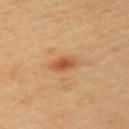Assessment:
Imaged during a routine full-body skin examination; the lesion was not biopsied and no histopathology is available.
Clinical summary:
A male subject roughly 40 years of age. On the upper back. This image is a 15 mm lesion crop taken from a total-body photograph.